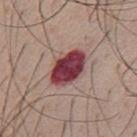Imaged during a routine full-body skin examination; the lesion was not biopsied and no histopathology is available.
Located on the chest.
Captured under white-light illumination.
A male subject, aged approximately 55.
The lesion's longest dimension is about 5 mm.
A region of skin cropped from a whole-body photographic capture, roughly 15 mm wide.
Automated image analysis of the tile measured a border-irregularity index near 1.5/10, internal color variation of about 5 on a 0–10 scale, and peripheral color asymmetry of about 1.5. The software also gave a classifier nevus-likeness of about 0/100 and a lesion-detection confidence of about 100/100.